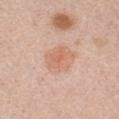Assessment: This lesion was catalogued during total-body skin photography and was not selected for biopsy. Context: A lesion tile, about 15 mm wide, cut from a 3D total-body photograph. This is a white-light tile. The subject is a female aged 38–42. Located on the chest. Approximately 4.5 mm at its widest.